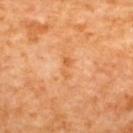Q: Is there a histopathology result?
A: total-body-photography surveillance lesion; no biopsy
Q: What are the patient's age and sex?
A: female, aged 63–67
Q: Lesion size?
A: about 3 mm
Q: Lesion location?
A: the upper back
Q: What lighting was used for the tile?
A: cross-polarized
Q: Automated lesion metrics?
A: an area of roughly 3 mm², a shape eccentricity near 0.9, and a symmetry-axis asymmetry near 0.4; a mean CIELAB color near L≈61 a*≈27 b*≈45, roughly 7 lightness units darker than nearby skin, and a normalized border contrast of about 5.5; a border-irregularity rating of about 5/10, a color-variation rating of about 0.5/10, and peripheral color asymmetry of about 0
Q: How was this image acquired?
A: 15 mm crop, total-body photography